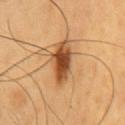The lesion is located on the chest. A male patient, aged 58–62. A close-up tile cropped from a whole-body skin photograph, about 15 mm across. Automated image analysis of the tile measured a mean CIELAB color near L≈49 a*≈24 b*≈39, roughly 18 lightness units darker than nearby skin, and a normalized lesion–skin contrast near 12. The software also gave a border-irregularity index near 3/10, a within-lesion color-variation index near 5/10, and a peripheral color-asymmetry measure near 1. It also reported a nevus-likeness score of about 95/100 and lesion-presence confidence of about 100/100.